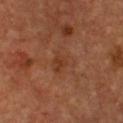follow-up = total-body-photography surveillance lesion; no biopsy
patient = male, about 50 years old
imaging modality = ~15 mm crop, total-body skin-cancer survey
anatomic site = the chest